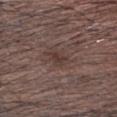workup: catalogued during a skin exam; not biopsied
anatomic site: the head or neck
size: about 2.5 mm
acquisition: total-body-photography crop, ~15 mm field of view
lighting: white-light illumination
patient: male, aged 43 to 47
automated metrics: a lesion area of about 3 mm², an outline eccentricity of about 0.75 (0 = round, 1 = elongated), and a symmetry-axis asymmetry near 0.3; a border-irregularity rating of about 3/10, a color-variation rating of about 2/10, and a peripheral color-asymmetry measure near 1; a detector confidence of about 100 out of 100 that the crop contains a lesion